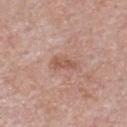The lesion was photographed on a routine skin check and not biopsied; there is no pathology result. A male subject aged around 55. A 15 mm close-up extracted from a 3D total-body photography capture. The recorded lesion diameter is about 3 mm. On the chest. The tile uses white-light illumination.